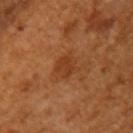notes: no biopsy performed (imaged during a skin exam) | patient: female, approximately 55 years of age | diameter: ~2.5 mm (longest diameter) | automated metrics: an outline eccentricity of about 0.35 (0 = round, 1 = elongated); an average lesion color of about L≈40 a*≈26 b*≈38 (CIELAB), about 7 CIELAB-L* units darker than the surrounding skin, and a normalized lesion–skin contrast near 6; a lesion-detection confidence of about 100/100 | image: ~15 mm tile from a whole-body skin photo | site: the left upper arm.